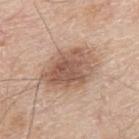Impression: Part of a total-body skin-imaging series; this lesion was reviewed on a skin check and was not flagged for biopsy. Context: Imaged with white-light lighting. This image is a 15 mm lesion crop taken from a total-body photograph. Longest diameter approximately 6.5 mm. From the right upper arm. A male patient, aged 78–82.